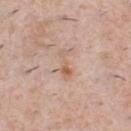{"biopsy_status": "not biopsied; imaged during a skin examination", "patient": {"sex": "male", "age_approx": 40}, "site": "chest", "lighting": "white-light", "image": {"source": "total-body photography crop", "field_of_view_mm": 15}}